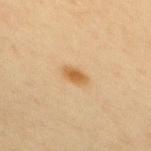workup = total-body-photography surveillance lesion; no biopsy | tile lighting = cross-polarized | acquisition = ~15 mm tile from a whole-body skin photo | body site = the upper back | TBP lesion metrics = a border-irregularity rating of about 1.5/10, a color-variation rating of about 1.5/10, and radial color variation of about 0.5; an automated nevus-likeness rating near 95 out of 100 and a detector confidence of about 100 out of 100 that the crop contains a lesion | patient = female, roughly 45 years of age.